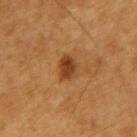Q: Is there a histopathology result?
A: no biopsy performed (imaged during a skin exam)
Q: Where on the body is the lesion?
A: the left upper arm
Q: Illumination type?
A: cross-polarized illumination
Q: What is the lesion's diameter?
A: about 3 mm
Q: Patient demographics?
A: male, aged approximately 60
Q: How was this image acquired?
A: ~15 mm tile from a whole-body skin photo